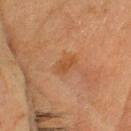The lesion was tiled from a total-body skin photograph and was not biopsied.
Cropped from a total-body skin-imaging series; the visible field is about 15 mm.
An algorithmic analysis of the crop reported a lesion area of about 3 mm², a shape eccentricity near 0.85, and a shape-asymmetry score of about 0.35 (0 = symmetric). It also reported a mean CIELAB color near L≈44 a*≈22 b*≈35, roughly 6 lightness units darker than nearby skin, and a lesion-to-skin contrast of about 6 (normalized; higher = more distinct). The analysis additionally found a classifier nevus-likeness of about 0/100 and a lesion-detection confidence of about 100/100.
A female patient, approximately 55 years of age.
The tile uses cross-polarized illumination.
From the head or neck.